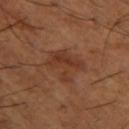Recorded during total-body skin imaging; not selected for excision or biopsy.